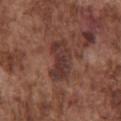Findings:
* workup: no biopsy performed (imaged during a skin exam)
* automated lesion analysis: a shape eccentricity near 0.85 and a symmetry-axis asymmetry near 0.25; a mean CIELAB color near L≈36 a*≈20 b*≈22, roughly 9 lightness units darker than nearby skin, and a normalized lesion–skin contrast near 8.5; border irregularity of about 3 on a 0–10 scale, a color-variation rating of about 4/10, and peripheral color asymmetry of about 1.5; an automated nevus-likeness rating near 0 out of 100 and a lesion-detection confidence of about 95/100
* lesion size: ≈5 mm
* image source: 15 mm crop, total-body photography
* tile lighting: white-light illumination
* location: the chest
* subject: male, in their mid-70s